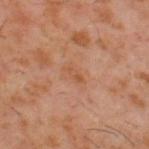Assessment: Captured during whole-body skin photography for melanoma surveillance; the lesion was not biopsied. Acquisition and patient details: The subject is a male aged 58–62. The tile uses cross-polarized illumination. The total-body-photography lesion software estimated a lesion color around L≈49 a*≈22 b*≈33 in CIELAB and a lesion–skin lightness drop of about 6. The analysis additionally found an automated nevus-likeness rating near 0 out of 100 and lesion-presence confidence of about 100/100. The lesion's longest dimension is about 2.5 mm. The lesion is located on the upper back. A roughly 15 mm field-of-view crop from a total-body skin photograph.